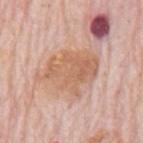Assessment:
Captured during whole-body skin photography for melanoma surveillance; the lesion was not biopsied.
Clinical summary:
On the mid back. The patient is a male roughly 80 years of age. Cropped from a total-body skin-imaging series; the visible field is about 15 mm.